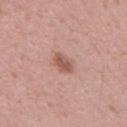- follow-up — imaged on a skin check; not biopsied
- lighting — white-light illumination
- body site — the abdomen
- lesion size — about 3 mm
- patient — male, in their mid- to late 50s
- imaging modality — ~15 mm crop, total-body skin-cancer survey
- automated metrics — an area of roughly 4.5 mm² and a shape eccentricity near 0.75; border irregularity of about 2.5 on a 0–10 scale and a within-lesion color-variation index near 3/10